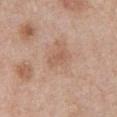  biopsy_status: not biopsied; imaged during a skin examination
  lesion_size:
    long_diameter_mm_approx: 2.5
  lighting: white-light
  image:
    source: total-body photography crop
    field_of_view_mm: 15
  site: front of the torso
  automated_metrics:
    area_mm2_approx: 3.5
    eccentricity: 0.85
    shape_asymmetry: 0.35
    vs_skin_darker_L: 7.0
    vs_skin_contrast_norm: 5.0
    border_irregularity_0_10: 3.0
    color_variation_0_10: 1.0
    peripheral_color_asymmetry: 0.5
  patient:
    sex: male
    age_approx: 70